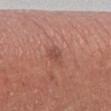Assessment:
Part of a total-body skin-imaging series; this lesion was reviewed on a skin check and was not flagged for biopsy.
Acquisition and patient details:
Approximately 2.5 mm at its widest. An algorithmic analysis of the crop reported an eccentricity of roughly 0.65 and a shape-asymmetry score of about 0.4 (0 = symmetric). It also reported border irregularity of about 3.5 on a 0–10 scale and internal color variation of about 2.5 on a 0–10 scale. Cropped from a total-body skin-imaging series; the visible field is about 15 mm. From the left lower leg. A male subject, approximately 70 years of age.